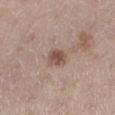lighting: white-light illumination
acquisition: ~15 mm tile from a whole-body skin photo
patient: female, aged 38 to 42
location: the leg